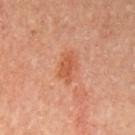Q: How was this image acquired?
A: total-body-photography crop, ~15 mm field of view
Q: Lesion location?
A: the left upper arm
Q: Who is the patient?
A: male, aged 63 to 67